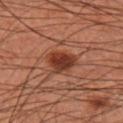Q: Lesion location?
A: the left forearm
Q: Illumination type?
A: cross-polarized
Q: What are the patient's age and sex?
A: male, aged 58 to 62
Q: How was this image acquired?
A: 15 mm crop, total-body photography
Q: How large is the lesion?
A: about 3.5 mm